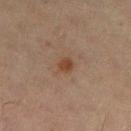Q: Was a biopsy performed?
A: no biopsy performed (imaged during a skin exam)
Q: Automated lesion metrics?
A: a lesion area of about 3.5 mm², an eccentricity of roughly 0.45, and a shape-asymmetry score of about 0.2 (0 = symmetric); a mean CIELAB color near L≈38 a*≈17 b*≈27; border irregularity of about 1.5 on a 0–10 scale and a color-variation rating of about 1.5/10; an automated nevus-likeness rating near 75 out of 100 and lesion-presence confidence of about 100/100
Q: What is the imaging modality?
A: 15 mm crop, total-body photography
Q: Where on the body is the lesion?
A: the left thigh
Q: Lesion size?
A: about 2 mm
Q: Who is the patient?
A: female, aged 53 to 57